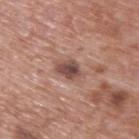Q: Is there a histopathology result?
A: catalogued during a skin exam; not biopsied
Q: Illumination type?
A: white-light illumination
Q: What are the patient's age and sex?
A: male, approximately 70 years of age
Q: How was this image acquired?
A: 15 mm crop, total-body photography
Q: What is the anatomic site?
A: the back
Q: Automated lesion metrics?
A: a lesion area of about 4.5 mm², an outline eccentricity of about 0.6 (0 = round, 1 = elongated), and a shape-asymmetry score of about 0.3 (0 = symmetric); radial color variation of about 1.5; a classifier nevus-likeness of about 45/100